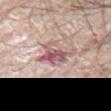• follow-up: imaged on a skin check; not biopsied
• size: ≈4 mm
• anatomic site: the left upper arm
• illumination: white-light
• patient: male, aged approximately 65
• image source: ~15 mm crop, total-body skin-cancer survey